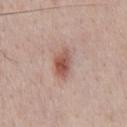The recorded lesion diameter is about 4 mm.
The tile uses white-light illumination.
The lesion is on the chest.
The lesion-visualizer software estimated a lesion area of about 6.5 mm² and an eccentricity of roughly 0.85. The software also gave a mean CIELAB color near L≈54 a*≈23 b*≈26 and a normalized border contrast of about 8.5. It also reported border irregularity of about 2.5 on a 0–10 scale and internal color variation of about 4.5 on a 0–10 scale. The analysis additionally found a nevus-likeness score of about 95/100 and lesion-presence confidence of about 100/100.
A region of skin cropped from a whole-body photographic capture, roughly 15 mm wide.
A male subject approximately 40 years of age.